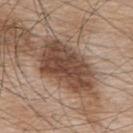{
  "biopsy_status": "not biopsied; imaged during a skin examination"
}